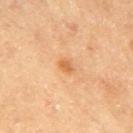biopsy status = no biopsy performed (imaged during a skin exam)
imaging modality = 15 mm crop, total-body photography
lesion diameter = ≈2 mm
subject = female, aged around 65
illumination = cross-polarized illumination
anatomic site = the arm
automated lesion analysis = a mean CIELAB color near L≈65 a*≈25 b*≈44; a classifier nevus-likeness of about 25/100 and a detector confidence of about 100 out of 100 that the crop contains a lesion